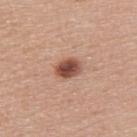workup: catalogued during a skin exam; not biopsied
imaging modality: 15 mm crop, total-body photography
patient: female, aged 48 to 52
site: the upper back